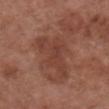Clinical impression: Imaged during a routine full-body skin examination; the lesion was not biopsied and no histopathology is available. Clinical summary: Automated image analysis of the tile measured an area of roughly 14 mm², a shape eccentricity near 0.85, and two-axis asymmetry of about 0.55. The software also gave an average lesion color of about L≈41 a*≈23 b*≈27 (CIELAB), roughly 7 lightness units darker than nearby skin, and a lesion-to-skin contrast of about 6 (normalized; higher = more distinct). And it measured a border-irregularity rating of about 7.5/10 and radial color variation of about 1. And it measured an automated nevus-likeness rating near 0 out of 100 and a detector confidence of about 100 out of 100 that the crop contains a lesion. About 6.5 mm across. From the chest. Imaged with white-light lighting. A female subject, aged approximately 75. A lesion tile, about 15 mm wide, cut from a 3D total-body photograph.